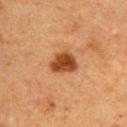Clinical impression: Part of a total-body skin-imaging series; this lesion was reviewed on a skin check and was not flagged for biopsy. Context: Captured under cross-polarized illumination. A 15 mm close-up extracted from a 3D total-body photography capture. A female patient aged 53 to 57. On the back.